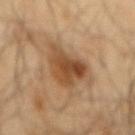Recorded during total-body skin imaging; not selected for excision or biopsy.
The lesion is located on the mid back.
Cropped from a whole-body photographic skin survey; the tile spans about 15 mm.
The tile uses cross-polarized illumination.
The lesion-visualizer software estimated an eccentricity of roughly 0.7 and two-axis asymmetry of about 0.4. The analysis additionally found roughly 11 lightness units darker than nearby skin and a normalized lesion–skin contrast near 8.5. The analysis additionally found a border-irregularity rating of about 4.5/10 and internal color variation of about 5.5 on a 0–10 scale. And it measured an automated nevus-likeness rating near 90 out of 100.
About 6 mm across.
A male patient aged around 65.